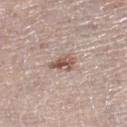Findings:
– biopsy status · catalogued during a skin exam; not biopsied
– site · the right lower leg
– subject · female, aged approximately 65
– image · total-body-photography crop, ~15 mm field of view